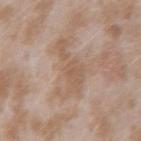Imaged during a routine full-body skin examination; the lesion was not biopsied and no histopathology is available. On the left forearm. Imaged with white-light lighting. Cropped from a total-body skin-imaging series; the visible field is about 15 mm. Automated tile analysis of the lesion measured a lesion color around L≈57 a*≈16 b*≈29 in CIELAB and about 7 CIELAB-L* units darker than the surrounding skin. The software also gave border irregularity of about 8.5 on a 0–10 scale, a color-variation rating of about 2/10, and a peripheral color-asymmetry measure near 0.5. A female subject in their mid-20s.